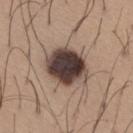This lesion was catalogued during total-body skin photography and was not selected for biopsy. A male subject, approximately 65 years of age. A close-up tile cropped from a whole-body skin photograph, about 15 mm across. Located on the leg. The lesion's longest dimension is about 6 mm. The tile uses white-light illumination.